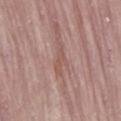biopsy status = imaged on a skin check; not biopsied
image = total-body-photography crop, ~15 mm field of view
body site = the lower back
tile lighting = white-light illumination
lesion size = ≈3 mm
subject = female, in their mid- to late 60s